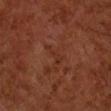This lesion was catalogued during total-body skin photography and was not selected for biopsy. This is a cross-polarized tile. The lesion's longest dimension is about 3 mm. A 15 mm crop from a total-body photograph taken for skin-cancer surveillance. The subject is a male about 60 years old. On the right forearm.